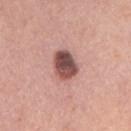Q: Was this lesion biopsied?
A: total-body-photography surveillance lesion; no biopsy
Q: Where on the body is the lesion?
A: the right upper arm
Q: What is the lesion's diameter?
A: ~3.5 mm (longest diameter)
Q: Illumination type?
A: white-light illumination
Q: Patient demographics?
A: female, aged 58 to 62
Q: What is the imaging modality?
A: ~15 mm crop, total-body skin-cancer survey